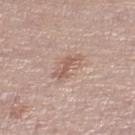Q: Is there a histopathology result?
A: imaged on a skin check; not biopsied
Q: Where on the body is the lesion?
A: the left thigh
Q: Patient demographics?
A: female, in their mid- to late 60s
Q: What kind of image is this?
A: ~15 mm crop, total-body skin-cancer survey